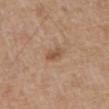Assessment:
Recorded during total-body skin imaging; not selected for excision or biopsy.
Clinical summary:
A close-up tile cropped from a whole-body skin photograph, about 15 mm across. Automated tile analysis of the lesion measured a lesion color around L≈53 a*≈19 b*≈32 in CIELAB, roughly 9 lightness units darker than nearby skin, and a normalized border contrast of about 6.5. The software also gave a border-irregularity index near 3/10, internal color variation of about 2.5 on a 0–10 scale, and peripheral color asymmetry of about 1. And it measured an automated nevus-likeness rating near 15 out of 100 and a detector confidence of about 100 out of 100 that the crop contains a lesion. The subject is a male aged around 70. Located on the abdomen. The lesion's longest dimension is about 3 mm.